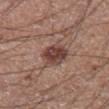| field | value |
|---|---|
| biopsy status | total-body-photography surveillance lesion; no biopsy |
| imaging modality | 15 mm crop, total-body photography |
| lighting | white-light illumination |
| subject | male, aged 73–77 |
| site | the abdomen |
| automated metrics | a mean CIELAB color near L≈42 a*≈20 b*≈23, roughly 12 lightness units darker than nearby skin, and a lesion-to-skin contrast of about 9.5 (normalized; higher = more distinct); lesion-presence confidence of about 100/100 |
| size | ≈3.5 mm |